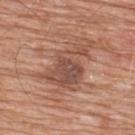Case summary:
* follow-up · catalogued during a skin exam; not biopsied
* TBP lesion metrics · an area of roughly 17 mm², a shape eccentricity near 0.8, and a symmetry-axis asymmetry near 0.45; about 10 CIELAB-L* units darker than the surrounding skin and a lesion-to-skin contrast of about 7 (normalized; higher = more distinct); a border-irregularity index near 8/10 and radial color variation of about 1.5
* size · about 7 mm
* body site · the upper back
* tile lighting · white-light illumination
* patient · male, aged 78–82
* image source · 15 mm crop, total-body photography